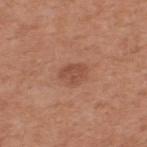Clinical impression:
Recorded during total-body skin imaging; not selected for excision or biopsy.
Image and clinical context:
A male subject, aged 53–57. This image is a 15 mm lesion crop taken from a total-body photograph. Measured at roughly 3 mm in maximum diameter. This is a white-light tile. From the upper back.